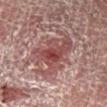workup: total-body-photography surveillance lesion; no biopsy | patient: male, roughly 55 years of age | lighting: cross-polarized illumination | acquisition: total-body-photography crop, ~15 mm field of view | size: ~6 mm (longest diameter) | location: the right lower leg.